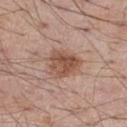Recorded during total-body skin imaging; not selected for excision or biopsy.
Longest diameter approximately 4 mm.
The tile uses white-light illumination.
The patient is a male aged approximately 55.
A roughly 15 mm field-of-view crop from a total-body skin photograph.
From the right thigh.